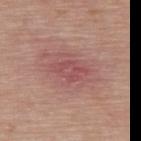The lesion was tiled from a total-body skin photograph and was not biopsied.
Located on the upper back.
The lesion's longest dimension is about 4 mm.
A male patient in their mid- to late 80s or older.
A region of skin cropped from a whole-body photographic capture, roughly 15 mm wide.
Automated tile analysis of the lesion measured an average lesion color of about L≈50 a*≈27 b*≈22 (CIELAB), a lesion–skin lightness drop of about 7, and a normalized border contrast of about 5.5.
Captured under white-light illumination.